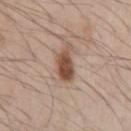Impression: Captured during whole-body skin photography for melanoma surveillance; the lesion was not biopsied. Clinical summary: The lesion is on the left upper arm. Approximately 3.5 mm at its widest. A 15 mm close-up tile from a total-body photography series done for melanoma screening. Automated tile analysis of the lesion measured an eccentricity of roughly 0.75 and two-axis asymmetry of about 0.4. And it measured a within-lesion color-variation index near 3/10 and peripheral color asymmetry of about 1. A male patient aged 68 to 72.